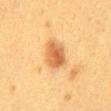biopsy status = imaged on a skin check; not biopsied | image = total-body-photography crop, ~15 mm field of view | body site = the mid back | subject = female, in their 40s | diameter = about 3.5 mm | illumination = cross-polarized.